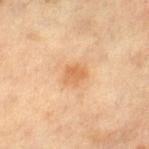follow-up: no biopsy performed (imaged during a skin exam)
lighting: cross-polarized illumination
imaging modality: 15 mm crop, total-body photography
diameter: ≈2.5 mm
anatomic site: the right thigh
automated metrics: an average lesion color of about L≈57 a*≈21 b*≈37 (CIELAB), a lesion–skin lightness drop of about 8, and a lesion-to-skin contrast of about 6.5 (normalized; higher = more distinct); border irregularity of about 2.5 on a 0–10 scale, a within-lesion color-variation index near 2/10, and radial color variation of about 0.5; an automated nevus-likeness rating near 55 out of 100 and lesion-presence confidence of about 100/100
subject: female, aged 53 to 57